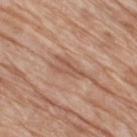Notes:
• workup — imaged on a skin check; not biopsied
• imaging modality — total-body-photography crop, ~15 mm field of view
• location — the right thigh
• tile lighting — white-light illumination
• diameter — ≈4 mm
• subject — female, aged around 75